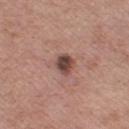- biopsy status — total-body-photography surveillance lesion; no biopsy
- body site — the left thigh
- lighting — white-light
- acquisition — total-body-photography crop, ~15 mm field of view
- patient — female, aged 73–77
- diameter — ~2.5 mm (longest diameter)